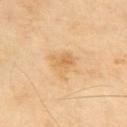Impression: Recorded during total-body skin imaging; not selected for excision or biopsy. Clinical summary: Measured at roughly 3.5 mm in maximum diameter. On the upper back. A male patient, aged approximately 65. Captured under cross-polarized illumination. A roughly 15 mm field-of-view crop from a total-body skin photograph.